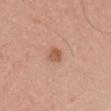Located on the head or neck. A female subject aged 38 to 42. A 15 mm close-up tile from a total-body photography series done for melanoma screening.